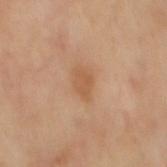Clinical impression: The lesion was photographed on a routine skin check and not biopsied; there is no pathology result. Clinical summary: Cropped from a whole-body photographic skin survey; the tile spans about 15 mm. The lesion is located on the mid back. Imaged with cross-polarized lighting. Measured at roughly 3 mm in maximum diameter.